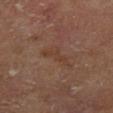workup — catalogued during a skin exam; not biopsied
patient — female, roughly 75 years of age
location — the right lower leg
acquisition — 15 mm crop, total-body photography